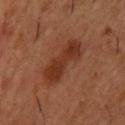Cropped from a whole-body photographic skin survey; the tile spans about 15 mm. The recorded lesion diameter is about 6.5 mm. Automated image analysis of the tile measured a footprint of about 13 mm², an outline eccentricity of about 0.95 (0 = round, 1 = elongated), and a symmetry-axis asymmetry near 0.2. The tile uses cross-polarized illumination. The subject is a male in their mid- to late 50s. The lesion is on the upper back.